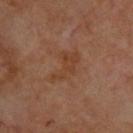workup: imaged on a skin check; not biopsied
body site: the upper back
illumination: cross-polarized
size: ≈4.5 mm
patient: aged around 65
acquisition: ~15 mm tile from a whole-body skin photo
image-analysis metrics: a lesion area of about 8 mm²; border irregularity of about 6 on a 0–10 scale and peripheral color asymmetry of about 0.5; a nevus-likeness score of about 0/100 and lesion-presence confidence of about 100/100